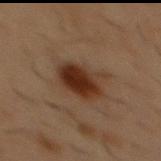  lesion_size:
    long_diameter_mm_approx: 4.5
  image:
    source: total-body photography crop
    field_of_view_mm: 15
  patient:
    sex: male
    age_approx: 55
  site: chest